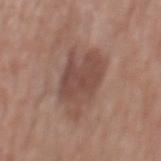Q: Was a biopsy performed?
A: imaged on a skin check; not biopsied
Q: Where on the body is the lesion?
A: the mid back
Q: Who is the patient?
A: male, aged around 65
Q: What is the lesion's diameter?
A: about 6.5 mm
Q: What kind of image is this?
A: ~15 mm crop, total-body skin-cancer survey